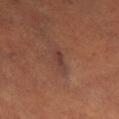follow-up: catalogued during a skin exam; not biopsied
imaging modality: ~15 mm tile from a whole-body skin photo
body site: the right lower leg
lesion diameter: ~3 mm (longest diameter)
automated metrics: a lesion area of about 3.5 mm², an eccentricity of roughly 0.9, and a symmetry-axis asymmetry near 0.3
patient: male, approximately 50 years of age
tile lighting: cross-polarized illumination